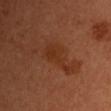Acquisition and patient details:
This is a cross-polarized tile. Measured at roughly 5.5 mm in maximum diameter. A female subject aged 48 to 52. A 15 mm close-up extracted from a 3D total-body photography capture.